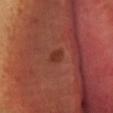Case summary:
* biopsy status: imaged on a skin check; not biopsied
* patient: female, roughly 30 years of age
* image: ~15 mm tile from a whole-body skin photo
* automated metrics: a lesion color around L≈36 a*≈27 b*≈30 in CIELAB, a lesion–skin lightness drop of about 8, and a normalized border contrast of about 6.5; lesion-presence confidence of about 100/100
* location: the head or neck